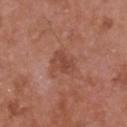Assessment: The lesion was photographed on a routine skin check and not biopsied; there is no pathology result. Background: Automated image analysis of the tile measured a footprint of about 6.5 mm², an eccentricity of roughly 0.75, and a symmetry-axis asymmetry near 0.3. And it measured a mean CIELAB color near L≈46 a*≈25 b*≈29, about 7 CIELAB-L* units darker than the surrounding skin, and a normalized lesion–skin contrast near 5.5. The analysis additionally found a peripheral color-asymmetry measure near 1. The analysis additionally found an automated nevus-likeness rating near 0 out of 100 and lesion-presence confidence of about 100/100. About 3.5 mm across. A male subject about 50 years old. Located on the head or neck. Cropped from a whole-body photographic skin survey; the tile spans about 15 mm. Imaged with white-light lighting.